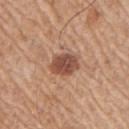This lesion was catalogued during total-body skin photography and was not selected for biopsy. The lesion is located on the right upper arm. The patient is a male about 65 years old. A 15 mm close-up extracted from a 3D total-body photography capture. This is a white-light tile. About 3.5 mm across. An algorithmic analysis of the crop reported a lesion area of about 7.5 mm² and a shape eccentricity near 0.55. The analysis additionally found a mean CIELAB color near L≈49 a*≈22 b*≈29, about 14 CIELAB-L* units darker than the surrounding skin, and a lesion-to-skin contrast of about 9.5 (normalized; higher = more distinct). The analysis additionally found a border-irregularity index near 1.5/10, a within-lesion color-variation index near 3/10, and peripheral color asymmetry of about 1.